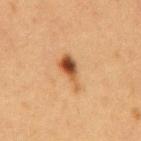notes = total-body-photography surveillance lesion; no biopsy
illumination = cross-polarized
lesion size = about 4.5 mm
patient = female, aged 38–42
acquisition = total-body-photography crop, ~15 mm field of view
body site = the mid back